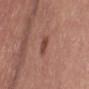{"lighting": "white-light", "lesion_size": {"long_diameter_mm_approx": 2.5}, "automated_metrics": {"area_mm2_approx": 3.5, "eccentricity": 0.75, "shape_asymmetry": 0.2}, "site": "leg", "patient": {"sex": "female", "age_approx": 55}, "image": {"source": "total-body photography crop", "field_of_view_mm": 15}}